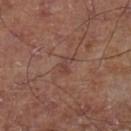workup: no biopsy performed (imaged during a skin exam); location: the left lower leg; size: about 2.5 mm; acquisition: 15 mm crop, total-body photography; illumination: cross-polarized.